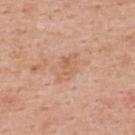Q: Was a biopsy performed?
A: imaged on a skin check; not biopsied
Q: Automated lesion metrics?
A: a lesion-detection confidence of about 100/100
Q: Illumination type?
A: white-light
Q: Patient demographics?
A: female, aged 48 to 52
Q: Lesion location?
A: the upper back
Q: How was this image acquired?
A: ~15 mm tile from a whole-body skin photo
Q: How large is the lesion?
A: ≈3.5 mm